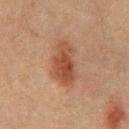The lesion was photographed on a routine skin check and not biopsied; there is no pathology result.
The lesion is on the chest.
Imaged with cross-polarized lighting.
Automated image analysis of the tile measured a shape eccentricity near 0.9 and a shape-asymmetry score of about 0.25 (0 = symmetric). And it measured an average lesion color of about L≈37 a*≈18 b*≈27 (CIELAB). The software also gave a border-irregularity index near 3/10, a color-variation rating of about 4/10, and radial color variation of about 1. The software also gave a nevus-likeness score of about 90/100.
Measured at roughly 6 mm in maximum diameter.
A male subject, approximately 60 years of age.
A region of skin cropped from a whole-body photographic capture, roughly 15 mm wide.